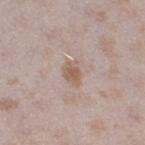notes = imaged on a skin check; not biopsied
location = the right thigh
imaging modality = ~15 mm tile from a whole-body skin photo
lighting = white-light illumination
subject = female, in their mid- to late 20s
lesion diameter = ≈2.5 mm